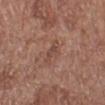Clinical impression:
The lesion was photographed on a routine skin check and not biopsied; there is no pathology result.
Context:
The lesion is located on the left lower leg. A close-up tile cropped from a whole-body skin photograph, about 15 mm across. Imaged with white-light lighting. The subject is a female about 80 years old. An algorithmic analysis of the crop reported a footprint of about 5 mm², an outline eccentricity of about 0.85 (0 = round, 1 = elongated), and a symmetry-axis asymmetry near 0.3. The analysis additionally found an average lesion color of about L≈47 a*≈21 b*≈25 (CIELAB), roughly 6 lightness units darker than nearby skin, and a normalized lesion–skin contrast near 5. It also reported lesion-presence confidence of about 100/100. About 3.5 mm across.